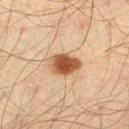| feature | finding |
|---|---|
| anatomic site | the left thigh |
| imaging modality | ~15 mm tile from a whole-body skin photo |
| image-analysis metrics | an area of roughly 9 mm² and a symmetry-axis asymmetry near 0.15; an average lesion color of about L≈45 a*≈20 b*≈31 (CIELAB) and a normalized border contrast of about 11.5; a border-irregularity rating of about 1.5/10 and a peripheral color-asymmetry measure near 1.5 |
| lesion diameter | about 4 mm |
| tile lighting | cross-polarized illumination |
| subject | male, aged 43–47 |